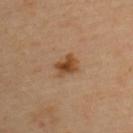Recorded during total-body skin imaging; not selected for excision or biopsy. Cropped from a whole-body photographic skin survey; the tile spans about 15 mm. About 3 mm across. Imaged with cross-polarized lighting. From the left upper arm. A female patient, approximately 40 years of age.